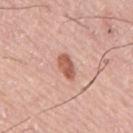notes: no biopsy performed (imaged during a skin exam) | subject: male, roughly 75 years of age | image source: ~15 mm tile from a whole-body skin photo | diameter: ≈3 mm | anatomic site: the mid back | tile lighting: white-light illumination.